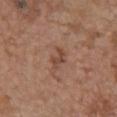illumination = white-light; image = 15 mm crop, total-body photography; diameter = ~2.5 mm (longest diameter); image-analysis metrics = a border-irregularity index near 5/10 and a color-variation rating of about 0/10; patient = female, aged approximately 65; location = the front of the torso.